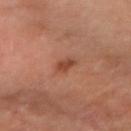Findings:
* workup — total-body-photography surveillance lesion; no biopsy
* subject — female, aged 43 to 47
* image-analysis metrics — a lesion color around L≈46 a*≈27 b*≈33 in CIELAB
* acquisition — ~15 mm tile from a whole-body skin photo
* lighting — cross-polarized
* diameter — ≈2 mm
* location — the right forearm